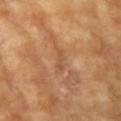notes: imaged on a skin check; not biopsied
site: the right upper arm
TBP lesion metrics: a footprint of about 2 mm², an eccentricity of roughly 0.95, and a shape-asymmetry score of about 0.45 (0 = symmetric); a lesion color around L≈52 a*≈23 b*≈36 in CIELAB, roughly 6 lightness units darker than nearby skin, and a normalized border contrast of about 4.5; lesion-presence confidence of about 90/100
image source: total-body-photography crop, ~15 mm field of view
tile lighting: cross-polarized illumination
diameter: about 2.5 mm
subject: female, aged 73–77